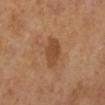notes: imaged on a skin check; not biopsied
automated metrics: an outline eccentricity of about 0.8 (0 = round, 1 = elongated) and a symmetry-axis asymmetry near 0.25; a border-irregularity index near 2.5/10 and peripheral color asymmetry of about 1; a nevus-likeness score of about 25/100
lesion size: ≈4 mm
body site: the leg
patient: female, aged 53–57
image: 15 mm crop, total-body photography
tile lighting: cross-polarized